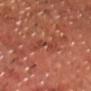The lesion was photographed on a routine skin check and not biopsied; there is no pathology result. The lesion's longest dimension is about 3.5 mm. A roughly 15 mm field-of-view crop from a total-body skin photograph. The patient is a male about 70 years old. From the chest.